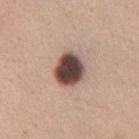Assessment: The lesion was tiled from a total-body skin photograph and was not biopsied. Image and clinical context: Automated tile analysis of the lesion measured a border-irregularity rating of about 1/10, internal color variation of about 6.5 on a 0–10 scale, and peripheral color asymmetry of about 1.5. The lesion's longest dimension is about 4 mm. A female subject, aged around 30. The lesion is located on the chest. Cropped from a whole-body photographic skin survey; the tile spans about 15 mm. Captured under white-light illumination.